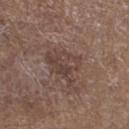  site: leg
  lesion_size:
    long_diameter_mm_approx: 4.5
  automated_metrics:
    area_mm2_approx: 10.0
    eccentricity: 0.65
    shape_asymmetry: 0.45
    vs_skin_darker_L: 7.0
    vs_skin_contrast_norm: 6.0
    color_variation_0_10: 5.5
    peripheral_color_asymmetry: 2.0
  image:
    source: total-body photography crop
    field_of_view_mm: 15
  patient:
    sex: male
    age_approx: 75